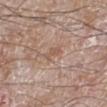{"patient": {"sex": "male", "age_approx": 60}, "automated_metrics": {"color_variation_0_10": 0.0, "peripheral_color_asymmetry": 0.0, "nevus_likeness_0_100": 0, "lesion_detection_confidence_0_100": 100}, "lesion_size": {"long_diameter_mm_approx": 2.5}, "image": {"source": "total-body photography crop", "field_of_view_mm": 15}, "site": "left lower leg"}